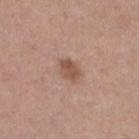follow-up — no biopsy performed (imaged during a skin exam) | lighting — white-light | body site — the left lower leg | patient — female, approximately 40 years of age | imaging modality — ~15 mm tile from a whole-body skin photo.